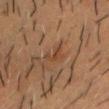Assessment:
Recorded during total-body skin imaging; not selected for excision or biopsy.
Context:
A 15 mm close-up extracted from a 3D total-body photography capture. The lesion is on the head or neck. Automated image analysis of the tile measured a lesion area of about 3.5 mm² and an outline eccentricity of about 0.8 (0 = round, 1 = elongated). It also reported an average lesion color of about L≈35 a*≈17 b*≈27 (CIELAB) and a normalized lesion–skin contrast near 5.5. It also reported a classifier nevus-likeness of about 0/100 and a detector confidence of about 90 out of 100 that the crop contains a lesion. A male subject, in their 60s. The tile uses cross-polarized illumination. About 3 mm across.